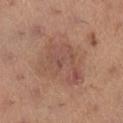workup = imaged on a skin check; not biopsied
lesion diameter = about 7 mm
anatomic site = the right lower leg
lighting = white-light
subject = female, in their mid- to late 60s
image source = ~15 mm crop, total-body skin-cancer survey